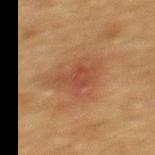Case summary:
• subject — female, aged around 70
• lesion size — ~4.5 mm (longest diameter)
• image-analysis metrics — a footprint of about 8.5 mm², an eccentricity of roughly 0.75, and two-axis asymmetry of about 0.35; a border-irregularity rating of about 4.5/10, internal color variation of about 2.5 on a 0–10 scale, and radial color variation of about 1
• anatomic site — the back
• image source — ~15 mm tile from a whole-body skin photo